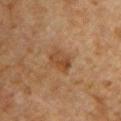Automated tile analysis of the lesion measured a lesion area of about 6.5 mm², an outline eccentricity of about 0.75 (0 = round, 1 = elongated), and two-axis asymmetry of about 0.25. And it measured a lesion–skin lightness drop of about 7 and a lesion-to-skin contrast of about 6.5 (normalized; higher = more distinct). The software also gave border irregularity of about 2.5 on a 0–10 scale, a color-variation rating of about 4/10, and radial color variation of about 1.5. Longest diameter approximately 3.5 mm. The tile uses cross-polarized illumination. A lesion tile, about 15 mm wide, cut from a 3D total-body photograph. The subject is a male aged approximately 60. From the chest.